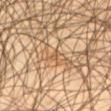notes: catalogued during a skin exam; not biopsied
location: the right thigh
size: about 2 mm
patient: male, in their mid-40s
automated metrics: a shape eccentricity near 0.9 and a shape-asymmetry score of about 0.35 (0 = symmetric); a nevus-likeness score of about 0/100 and lesion-presence confidence of about 0/100
tile lighting: cross-polarized
image source: ~15 mm tile from a whole-body skin photo